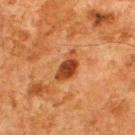biopsy status: imaged on a skin check; not biopsied
image: ~15 mm crop, total-body skin-cancer survey
anatomic site: the upper back
illumination: cross-polarized illumination
size: ≈4 mm
patient: male, aged around 80